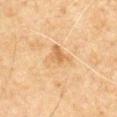{"biopsy_status": "not biopsied; imaged during a skin examination", "image": {"source": "total-body photography crop", "field_of_view_mm": 15}, "patient": {"sex": "male", "age_approx": 65}, "site": "chest"}